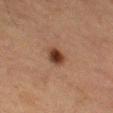Findings:
- biopsy status · imaged on a skin check; not biopsied